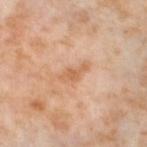Assessment:
Recorded during total-body skin imaging; not selected for excision or biopsy.
Context:
A female patient, about 55 years old. Longest diameter approximately 3.5 mm. The lesion-visualizer software estimated about 8 CIELAB-L* units darker than the surrounding skin and a normalized lesion–skin contrast near 6. The analysis additionally found a within-lesion color-variation index near 1.5/10 and a peripheral color-asymmetry measure near 0.5. Cropped from a total-body skin-imaging series; the visible field is about 15 mm. On the left thigh. Captured under cross-polarized illumination.